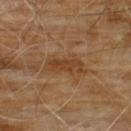| field | value |
|---|---|
| notes | total-body-photography surveillance lesion; no biopsy |
| subject | male, aged around 60 |
| illumination | cross-polarized illumination |
| TBP lesion metrics | a lesion area of about 8.5 mm², an outline eccentricity of about 0.85 (0 = round, 1 = elongated), and two-axis asymmetry of about 0.3; a lesion color around L≈40 a*≈19 b*≈33 in CIELAB, about 7 CIELAB-L* units darker than the surrounding skin, and a lesion-to-skin contrast of about 6.5 (normalized; higher = more distinct) |
| image source | 15 mm crop, total-body photography |
| anatomic site | the chest |